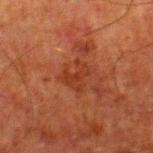Q: Is there a histopathology result?
A: catalogued during a skin exam; not biopsied
Q: Illumination type?
A: cross-polarized illumination
Q: Where on the body is the lesion?
A: the right lower leg
Q: Who is the patient?
A: male, aged around 80
Q: What kind of image is this?
A: 15 mm crop, total-body photography